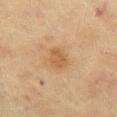A female patient, roughly 55 years of age. The lesion is located on the left thigh. This image is a 15 mm lesion crop taken from a total-body photograph. Measured at roughly 2.5 mm in maximum diameter.